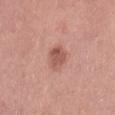{
  "biopsy_status": "not biopsied; imaged during a skin examination",
  "patient": {
    "sex": "female",
    "age_approx": 40
  },
  "lesion_size": {
    "long_diameter_mm_approx": 3.5
  },
  "site": "right thigh",
  "image": {
    "source": "total-body photography crop",
    "field_of_view_mm": 15
  }
}